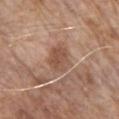Impression:
Part of a total-body skin-imaging series; this lesion was reviewed on a skin check and was not flagged for biopsy.
Image and clinical context:
The lesion is on the chest. Automated tile analysis of the lesion measured a lesion area of about 8.5 mm² and a shape eccentricity near 0.65. Cropped from a whole-body photographic skin survey; the tile spans about 15 mm. A male subject, aged approximately 80. Imaged with white-light lighting.